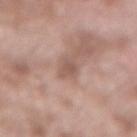{"patient": {"sex": "male", "age_approx": 55}, "site": "lower back", "image": {"source": "total-body photography crop", "field_of_view_mm": 15}, "lesion_size": {"long_diameter_mm_approx": 2.5}, "lighting": "white-light"}